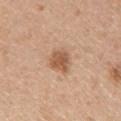No biopsy was performed on this lesion — it was imaged during a full skin examination and was not determined to be concerning. From the arm. A lesion tile, about 15 mm wide, cut from a 3D total-body photograph. The subject is a female aged 58 to 62. Longest diameter approximately 3 mm. The tile uses white-light illumination.